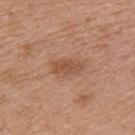Q: Is there a histopathology result?
A: imaged on a skin check; not biopsied
Q: Lesion location?
A: the left upper arm
Q: Patient demographics?
A: female, approximately 40 years of age
Q: Illumination type?
A: white-light illumination
Q: What is the imaging modality?
A: total-body-photography crop, ~15 mm field of view